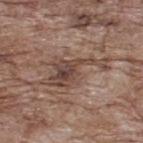location: the upper back
TBP lesion metrics: a footprint of about 13 mm², an outline eccentricity of about 0.9 (0 = round, 1 = elongated), and a symmetry-axis asymmetry near 0.6; an average lesion color of about L≈45 a*≈17 b*≈24 (CIELAB), a lesion–skin lightness drop of about 10, and a lesion-to-skin contrast of about 8 (normalized; higher = more distinct); a border-irregularity index near 9.5/10, internal color variation of about 5.5 on a 0–10 scale, and radial color variation of about 2; a classifier nevus-likeness of about 0/100 and a lesion-detection confidence of about 90/100
subject: male, aged 68 to 72
tile lighting: white-light illumination
image source: ~15 mm crop, total-body skin-cancer survey
diameter: about 7 mm